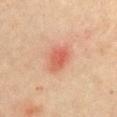Findings:
- workup: no biopsy performed (imaged during a skin exam)